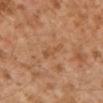Captured during whole-body skin photography for melanoma surveillance; the lesion was not biopsied.
Automated image analysis of the tile measured a border-irregularity index near 5.5/10 and a color-variation rating of about 1/10.
A close-up tile cropped from a whole-body skin photograph, about 15 mm across.
This is a cross-polarized tile.
Located on the left lower leg.
Measured at roughly 4.5 mm in maximum diameter.
The subject is a male aged approximately 30.